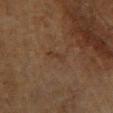Imaged during a routine full-body skin examination; the lesion was not biopsied and no histopathology is available. A male patient, approximately 60 years of age. On the right lower leg. A region of skin cropped from a whole-body photographic capture, roughly 15 mm wide. Measured at roughly 2.5 mm in maximum diameter. Automated image analysis of the tile measured an average lesion color of about L≈26 a*≈13 b*≈22 (CIELAB), a lesion–skin lightness drop of about 4, and a normalized border contrast of about 4.5. The analysis additionally found a color-variation rating of about 0/10 and a peripheral color-asymmetry measure near 0. It also reported a lesion-detection confidence of about 100/100. The tile uses cross-polarized illumination.